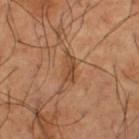{
  "biopsy_status": "not biopsied; imaged during a skin examination",
  "lesion_size": {
    "long_diameter_mm_approx": 3.5
  },
  "lighting": "cross-polarized",
  "image": {
    "source": "total-body photography crop",
    "field_of_view_mm": 15
  },
  "patient": {
    "sex": "male",
    "age_approx": 65
  },
  "site": "left thigh"
}